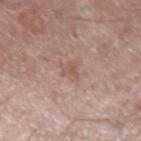No biopsy was performed on this lesion — it was imaged during a full skin examination and was not determined to be concerning. Cropped from a whole-body photographic skin survey; the tile spans about 15 mm. Captured under white-light illumination. The lesion-visualizer software estimated a lesion area of about 4 mm² and a shape-asymmetry score of about 0.4 (0 = symmetric). The analysis additionally found a border-irregularity index near 4.5/10 and a peripheral color-asymmetry measure near 0.5. It also reported a nevus-likeness score of about 0/100 and a detector confidence of about 100 out of 100 that the crop contains a lesion. Located on the arm. A male patient, roughly 60 years of age. The lesion's longest dimension is about 2.5 mm.